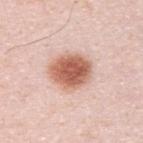The recorded lesion diameter is about 5 mm. A male patient aged approximately 35. The tile uses white-light illumination. A 15 mm close-up tile from a total-body photography series done for melanoma screening. From the upper back. The total-body-photography lesion software estimated a mean CIELAB color near L≈62 a*≈24 b*≈30. It also reported a border-irregularity rating of about 1/10 and a peripheral color-asymmetry measure near 1.5.